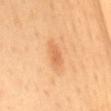<record>
<biopsy_status>not biopsied; imaged during a skin examination</biopsy_status>
<image>
  <source>total-body photography crop</source>
  <field_of_view_mm>15</field_of_view_mm>
</image>
<patient>
  <sex>female</sex>
  <age_approx>50</age_approx>
</patient>
<automated_metrics>
  <area_mm2_approx>3.5</area_mm2_approx>
  <eccentricity>0.9</eccentricity>
  <shape_asymmetry>0.35</shape_asymmetry>
  <border_irregularity_0_10>3.0</border_irregularity_0_10>
  <color_variation_0_10>1.0</color_variation_0_10>
  <peripheral_color_asymmetry>0.0</peripheral_color_asymmetry>
  <nevus_likeness_0_100>55</nevus_likeness_0_100>
  <lesion_detection_confidence_0_100>100</lesion_detection_confidence_0_100>
</automated_metrics>
<site>back</site>
</record>